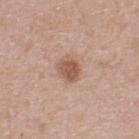Part of a total-body skin-imaging series; this lesion was reviewed on a skin check and was not flagged for biopsy.